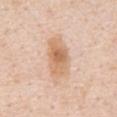follow-up: no biopsy performed (imaged during a skin exam); patient: male, in their 50s; body site: the abdomen; image source: ~15 mm tile from a whole-body skin photo.